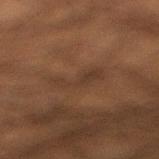Clinical impression: The lesion was photographed on a routine skin check and not biopsied; there is no pathology result. Background: A close-up tile cropped from a whole-body skin photograph, about 15 mm across. Automated image analysis of the tile measured a mean CIELAB color near L≈27 a*≈13 b*≈22, about 4 CIELAB-L* units darker than the surrounding skin, and a normalized lesion–skin contrast near 4.5. The software also gave a border-irregularity index near 5.5/10 and peripheral color asymmetry of about 0.5. Captured under cross-polarized illumination. A male subject aged 48 to 52. The lesion is located on the right lower leg. The lesion's longest dimension is about 4 mm.